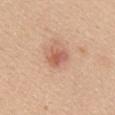<lesion>
<biopsy_status>not biopsied; imaged during a skin examination</biopsy_status>
<image>
  <source>total-body photography crop</source>
  <field_of_view_mm>15</field_of_view_mm>
</image>
<lighting>white-light</lighting>
<patient>
  <sex>female</sex>
  <age_approx>35</age_approx>
</patient>
<site>mid back</site>
<lesion_size>
  <long_diameter_mm_approx>2.5</long_diameter_mm_approx>
</lesion_size>
</lesion>